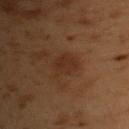Assessment:
Part of a total-body skin-imaging series; this lesion was reviewed on a skin check and was not flagged for biopsy.
Background:
The lesion is located on the chest. Measured at roughly 3 mm in maximum diameter. A roughly 15 mm field-of-view crop from a total-body skin photograph. A male subject, aged 53 to 57. The tile uses cross-polarized illumination.